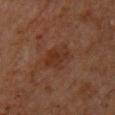Assessment: No biopsy was performed on this lesion — it was imaged during a full skin examination and was not determined to be concerning. Acquisition and patient details: A male subject aged 58 to 62. Located on the upper back. A 15 mm crop from a total-body photograph taken for skin-cancer surveillance.